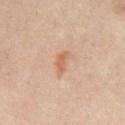notes — imaged on a skin check; not biopsied | lesion size — ~3 mm (longest diameter) | subject — about 55 years old | image-analysis metrics — an average lesion color of about L≈61 a*≈21 b*≈33 (CIELAB), about 8 CIELAB-L* units darker than the surrounding skin, and a lesion-to-skin contrast of about 6.5 (normalized; higher = more distinct); border irregularity of about 3.5 on a 0–10 scale and a color-variation rating of about 1.5/10 | tile lighting — cross-polarized illumination | site — the chest | acquisition — total-body-photography crop, ~15 mm field of view.